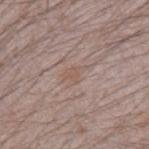notes: total-body-photography surveillance lesion; no biopsy | diameter: ~2.5 mm (longest diameter) | subject: male, about 25 years old | illumination: white-light illumination | acquisition: ~15 mm crop, total-body skin-cancer survey | anatomic site: the arm.